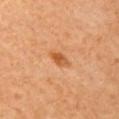{
  "biopsy_status": "not biopsied; imaged during a skin examination",
  "image": {
    "source": "total-body photography crop",
    "field_of_view_mm": 15
  },
  "patient": {
    "sex": "female",
    "age_approx": 65
  },
  "lesion_size": {
    "long_diameter_mm_approx": 3.0
  },
  "lighting": "cross-polarized",
  "site": "left upper arm"
}